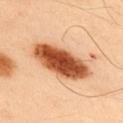Clinical impression:
The lesion was photographed on a routine skin check and not biopsied; there is no pathology result.
Image and clinical context:
About 7.5 mm across. Located on the upper back. Imaged with cross-polarized lighting. A male patient, aged 48 to 52. A close-up tile cropped from a whole-body skin photograph, about 15 mm across.